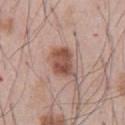biopsy status: imaged on a skin check; not biopsied | diameter: about 3.5 mm | site: the abdomen | automated lesion analysis: about 13 CIELAB-L* units darker than the surrounding skin and a lesion-to-skin contrast of about 9 (normalized; higher = more distinct); a border-irregularity rating of about 2.5/10 and radial color variation of about 1.5 | subject: male, in their mid-50s | tile lighting: white-light illumination | image source: ~15 mm crop, total-body skin-cancer survey.